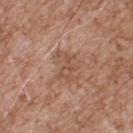Impression: Imaged during a routine full-body skin examination; the lesion was not biopsied and no histopathology is available. Background: A 15 mm crop from a total-body photograph taken for skin-cancer surveillance. Imaged with white-light lighting. Located on the upper back. Approximately 3.5 mm at its widest. The subject is a male aged 53 to 57. The lesion-visualizer software estimated a lesion area of about 6 mm², an eccentricity of roughly 0.8, and a symmetry-axis asymmetry near 0.5.